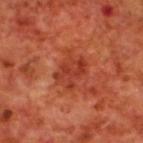The lesion was tiled from a total-body skin photograph and was not biopsied. A male subject aged 68 to 72. About 4 mm across. Captured under cross-polarized illumination. This image is a 15 mm lesion crop taken from a total-body photograph. The lesion is on the upper back.